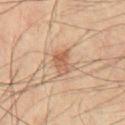Q: Was a biopsy performed?
A: imaged on a skin check; not biopsied
Q: What is the anatomic site?
A: the chest
Q: What lighting was used for the tile?
A: cross-polarized
Q: What is the imaging modality?
A: ~15 mm tile from a whole-body skin photo
Q: Patient demographics?
A: male, aged 58–62